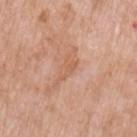Acquisition and patient details: About 2.5 mm across. A region of skin cropped from a whole-body photographic capture, roughly 15 mm wide. This is a white-light tile. A female subject, aged 68–72. On the left upper arm.